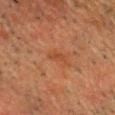No biopsy was performed on this lesion — it was imaged during a full skin examination and was not determined to be concerning.
The patient is a male about 60 years old.
On the head or neck.
A roughly 15 mm field-of-view crop from a total-body skin photograph.